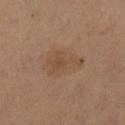<lesion>
  <lesion_size>
    <long_diameter_mm_approx>5.0</long_diameter_mm_approx>
  </lesion_size>
  <lighting>cross-polarized</lighting>
  <automated_metrics>
    <cielab_L>46</cielab_L>
    <cielab_a>16</cielab_a>
    <cielab_b>29</cielab_b>
    <vs_skin_darker_L>5.0</vs_skin_darker_L>
    <vs_skin_contrast_norm>5.0</vs_skin_contrast_norm>
    <border_irregularity_0_10>3.0</border_irregularity_0_10>
    <color_variation_0_10>2.0</color_variation_0_10>
    <peripheral_color_asymmetry>0.5</peripheral_color_asymmetry>
  </automated_metrics>
  <site>left lower leg</site>
  <patient>
    <sex>female</sex>
    <age_approx>35</age_approx>
  </patient>
  <image>
    <source>total-body photography crop</source>
    <field_of_view_mm>15</field_of_view_mm>
  </image>
</lesion>